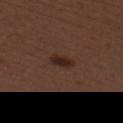Impression: Captured during whole-body skin photography for melanoma surveillance; the lesion was not biopsied. Clinical summary: The subject is a female aged around 50. The lesion is located on the back. A 15 mm close-up tile from a total-body photography series done for melanoma screening.